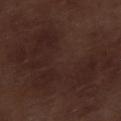{
  "biopsy_status": "not biopsied; imaged during a skin examination",
  "lesion_size": {
    "long_diameter_mm_approx": 15.5
  },
  "site": "right lower leg",
  "patient": {
    "sex": "male",
    "age_approx": 70
  },
  "image": {
    "source": "total-body photography crop",
    "field_of_view_mm": 15
  },
  "lighting": "white-light"
}